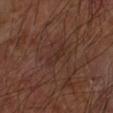Recorded during total-body skin imaging; not selected for excision or biopsy.
A 15 mm close-up extracted from a 3D total-body photography capture.
The lesion is on the left forearm.
A male patient aged approximately 70.